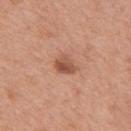Captured during whole-body skin photography for melanoma surveillance; the lesion was not biopsied.
The lesion is on the mid back.
The tile uses white-light illumination.
A roughly 15 mm field-of-view crop from a total-body skin photograph.
An algorithmic analysis of the crop reported a footprint of about 4.5 mm², an eccentricity of roughly 0.55, and two-axis asymmetry of about 0.25. The analysis additionally found a mean CIELAB color near L≈52 a*≈25 b*≈31, about 11 CIELAB-L* units darker than the surrounding skin, and a normalized border contrast of about 8. The analysis additionally found a border-irregularity rating of about 2/10, a within-lesion color-variation index near 4.5/10, and radial color variation of about 2. And it measured a classifier nevus-likeness of about 80/100 and a lesion-detection confidence of about 100/100.
The subject is a male in their 70s.